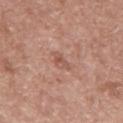No biopsy was performed on this lesion — it was imaged during a full skin examination and was not determined to be concerning.
A male patient roughly 75 years of age.
A close-up tile cropped from a whole-body skin photograph, about 15 mm across.
The lesion is located on the abdomen.